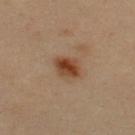Case summary:
- tile lighting · cross-polarized illumination
- automated lesion analysis · an area of roughly 6.5 mm², an outline eccentricity of about 0.75 (0 = round, 1 = elongated), and two-axis asymmetry of about 0.15; border irregularity of about 1.5 on a 0–10 scale and a within-lesion color-variation index near 6/10
- image source · total-body-photography crop, ~15 mm field of view
- patient · male, roughly 35 years of age
- location · the upper back
- size · ≈3.5 mm